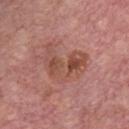- notes: catalogued during a skin exam; not biopsied
- anatomic site: the chest
- patient: male, in their mid-70s
- image: ~15 mm tile from a whole-body skin photo